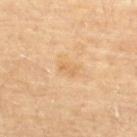Assessment: This lesion was catalogued during total-body skin photography and was not selected for biopsy. Image and clinical context: Cropped from a total-body skin-imaging series; the visible field is about 15 mm. The tile uses cross-polarized illumination. Automated tile analysis of the lesion measured a color-variation rating of about 2/10 and peripheral color asymmetry of about 0.5. From the upper back. A female patient aged around 80. The recorded lesion diameter is about 2.5 mm.